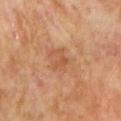The lesion was tiled from a total-body skin photograph and was not biopsied. A lesion tile, about 15 mm wide, cut from a 3D total-body photograph. Imaged with cross-polarized lighting. A male patient, aged around 65. The lesion is located on the arm. The total-body-photography lesion software estimated an average lesion color of about L≈51 a*≈23 b*≈35 (CIELAB) and a lesion–skin lightness drop of about 6. And it measured a color-variation rating of about 1/10 and peripheral color asymmetry of about 0.5. The lesion's longest dimension is about 2.5 mm.